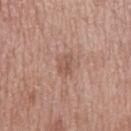Findings:
– workup · total-body-photography surveillance lesion; no biopsy
– TBP lesion metrics · a border-irregularity index near 3/10 and a peripheral color-asymmetry measure near 1
– lighting · white-light
– size · about 2.5 mm
– image · total-body-photography crop, ~15 mm field of view
– patient · male, about 65 years old
– anatomic site · the mid back